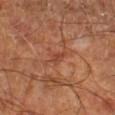Q: Was a biopsy performed?
A: imaged on a skin check; not biopsied
Q: How was this image acquired?
A: total-body-photography crop, ~15 mm field of view
Q: Lesion location?
A: the right lower leg
Q: Patient demographics?
A: aged approximately 65
Q: Illumination type?
A: cross-polarized illumination